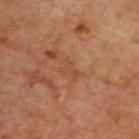Impression:
Recorded during total-body skin imaging; not selected for excision or biopsy.
Acquisition and patient details:
A male subject, about 70 years old. A region of skin cropped from a whole-body photographic capture, roughly 15 mm wide. The lesion is located on the upper back. The tile uses cross-polarized illumination.